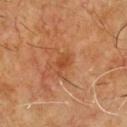Clinical impression:
No biopsy was performed on this lesion — it was imaged during a full skin examination and was not determined to be concerning.
Context:
Imaged with cross-polarized lighting. A roughly 15 mm field-of-view crop from a total-body skin photograph. Automated tile analysis of the lesion measured an average lesion color of about L≈43 a*≈25 b*≈36 (CIELAB), a lesion–skin lightness drop of about 7, and a normalized border contrast of about 6.5. The software also gave a border-irregularity rating of about 4.5/10 and internal color variation of about 1.5 on a 0–10 scale. The software also gave a nevus-likeness score of about 0/100 and a detector confidence of about 100 out of 100 that the crop contains a lesion. A male subject aged 58 to 62. Located on the chest. About 2.5 mm across.